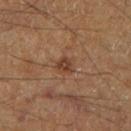No biopsy was performed on this lesion — it was imaged during a full skin examination and was not determined to be concerning. The lesion is on the right lower leg. The subject is a male in their 60s. Measured at roughly 2.5 mm in maximum diameter. Cropped from a total-body skin-imaging series; the visible field is about 15 mm. Captured under cross-polarized illumination.